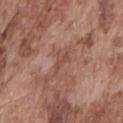{"biopsy_status": "not biopsied; imaged during a skin examination", "site": "mid back", "patient": {"sex": "male", "age_approx": 75}, "image": {"source": "total-body photography crop", "field_of_view_mm": 15}, "lesion_size": {"long_diameter_mm_approx": 3.0}}